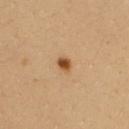biopsy status: total-body-photography surveillance lesion; no biopsy | lesion size: ~2 mm (longest diameter) | imaging modality: ~15 mm tile from a whole-body skin photo | anatomic site: the upper back | TBP lesion metrics: an area of roughly 3 mm² and a shape eccentricity near 0.6; a lesion color around L≈51 a*≈22 b*≈39 in CIELAB and roughly 14 lightness units darker than nearby skin; a classifier nevus-likeness of about 100/100 | patient: female, in their mid-30s.